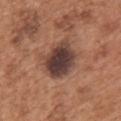biopsy status: no biopsy performed (imaged during a skin exam); imaging modality: ~15 mm tile from a whole-body skin photo; body site: the upper back; lighting: white-light illumination; patient: male, approximately 65 years of age; size: about 5 mm.